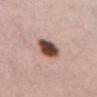Case summary:
• lighting — white-light illumination
• imaging modality — 15 mm crop, total-body photography
• patient — female, aged approximately 35
• lesion size — about 4 mm
• body site — the mid back